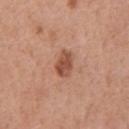The lesion is on the chest. A male subject aged 68–72. A lesion tile, about 15 mm wide, cut from a 3D total-body photograph.